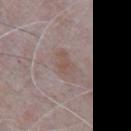workup: total-body-photography surveillance lesion; no biopsy | tile lighting: white-light | subject: male, in their mid- to late 60s | site: the chest | lesion size: ~4 mm (longest diameter) | automated metrics: a footprint of about 6.5 mm² and an outline eccentricity of about 0.8 (0 = round, 1 = elongated); a border-irregularity index near 3.5/10, internal color variation of about 3 on a 0–10 scale, and radial color variation of about 1; an automated nevus-likeness rating near 10 out of 100 and a detector confidence of about 85 out of 100 that the crop contains a lesion | imaging modality: total-body-photography crop, ~15 mm field of view.